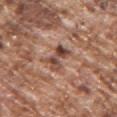Case summary:
• lesion diameter — about 3 mm
• anatomic site — the chest
• subject — male, approximately 75 years of age
• image — ~15 mm tile from a whole-body skin photo